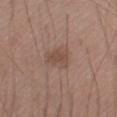This lesion was catalogued during total-body skin photography and was not selected for biopsy.